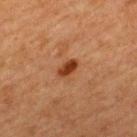<tbp_lesion>
<biopsy_status>not biopsied; imaged during a skin examination</biopsy_status>
<lesion_size>
  <long_diameter_mm_approx>2.5</long_diameter_mm_approx>
</lesion_size>
<site>upper back</site>
<patient>
  <sex>female</sex>
  <age_approx>40</age_approx>
</patient>
<image>
  <source>total-body photography crop</source>
  <field_of_view_mm>15</field_of_view_mm>
</image>
<lighting>cross-polarized</lighting>
</tbp_lesion>